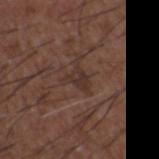From the upper back. A male subject aged approximately 50. The lesion's longest dimension is about 3 mm. A close-up tile cropped from a whole-body skin photograph, about 15 mm across. This is a white-light tile.